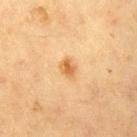Impression:
Recorded during total-body skin imaging; not selected for excision or biopsy.
Image and clinical context:
The subject is a female aged 53 to 57. From the right thigh. A 15 mm crop from a total-body photograph taken for skin-cancer surveillance.